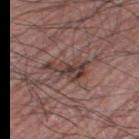The lesion was photographed on a routine skin check and not biopsied; there is no pathology result.
From the right thigh.
A 15 mm close-up tile from a total-body photography series done for melanoma screening.
The recorded lesion diameter is about 5.5 mm.
Automated image analysis of the tile measured a shape eccentricity near 0.9 and a shape-asymmetry score of about 0.55 (0 = symmetric). The analysis additionally found an average lesion color of about L≈39 a*≈18 b*≈20 (CIELAB), about 10 CIELAB-L* units darker than the surrounding skin, and a normalized lesion–skin contrast near 8. The analysis additionally found a border-irregularity index near 7/10, a color-variation rating of about 4.5/10, and a peripheral color-asymmetry measure near 1.5.
A male subject, about 75 years old.
Captured under white-light illumination.